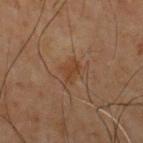- biopsy status — total-body-photography surveillance lesion; no biopsy
- tile lighting — cross-polarized illumination
- size — ≈2.5 mm
- TBP lesion metrics — a lesion area of about 3.5 mm², an outline eccentricity of about 0.75 (0 = round, 1 = elongated), and a shape-asymmetry score of about 0.45 (0 = symmetric); an average lesion color of about L≈30 a*≈16 b*≈25 (CIELAB), a lesion–skin lightness drop of about 5, and a lesion-to-skin contrast of about 6 (normalized; higher = more distinct); a border-irregularity rating of about 4/10, a color-variation rating of about 0.5/10, and a peripheral color-asymmetry measure near 0.5
- body site — the chest
- patient — male, approximately 70 years of age
- image — total-body-photography crop, ~15 mm field of view